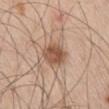Recorded during total-body skin imaging; not selected for excision or biopsy.
A male subject aged approximately 60.
On the leg.
A lesion tile, about 15 mm wide, cut from a 3D total-body photograph.